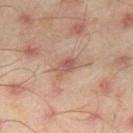This lesion was catalogued during total-body skin photography and was not selected for biopsy.
Automated tile analysis of the lesion measured a border-irregularity index near 3/10, a color-variation rating of about 4/10, and peripheral color asymmetry of about 1.5.
This image is a 15 mm lesion crop taken from a total-body photograph.
From the right thigh.
The recorded lesion diameter is about 3 mm.
The subject is a male approximately 45 years of age.
This is a cross-polarized tile.